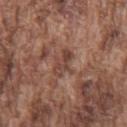biopsy_status: not biopsied; imaged during a skin examination